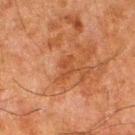Clinical impression:
No biopsy was performed on this lesion — it was imaged during a full skin examination and was not determined to be concerning.